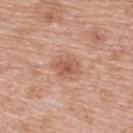The lesion was photographed on a routine skin check and not biopsied; there is no pathology result. From the back. The recorded lesion diameter is about 3 mm. Imaged with white-light lighting. A 15 mm close-up extracted from a 3D total-body photography capture. A female subject approximately 60 years of age.